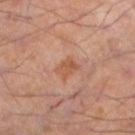The lesion was photographed on a routine skin check and not biopsied; there is no pathology result.
Located on the left lower leg.
Captured under cross-polarized illumination.
A male subject, aged around 55.
An algorithmic analysis of the crop reported a footprint of about 4 mm² and two-axis asymmetry of about 0.25.
A 15 mm close-up extracted from a 3D total-body photography capture.